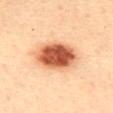Assessment:
The lesion was photographed on a routine skin check and not biopsied; there is no pathology result.
Clinical summary:
This image is a 15 mm lesion crop taken from a total-body photograph. A female subject, aged 33–37. On the mid back. The lesion's longest dimension is about 6.5 mm.